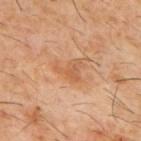Notes:
* notes: imaged on a skin check; not biopsied
* lesion size: ≈3.5 mm
* imaging modality: ~15 mm crop, total-body skin-cancer survey
* automated lesion analysis: an average lesion color of about L≈54 a*≈22 b*≈35 (CIELAB) and roughly 7 lightness units darker than nearby skin
* body site: the upper back
* illumination: cross-polarized illumination
* subject: male, aged approximately 60